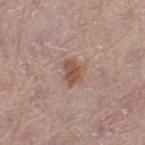Recorded during total-body skin imaging; not selected for excision or biopsy.
On the right thigh.
Imaged with white-light lighting.
Longest diameter approximately 3 mm.
A roughly 15 mm field-of-view crop from a total-body skin photograph.
A female subject, aged 63 to 67.
An algorithmic analysis of the crop reported border irregularity of about 3 on a 0–10 scale, internal color variation of about 2.5 on a 0–10 scale, and radial color variation of about 0.5. It also reported an automated nevus-likeness rating near 75 out of 100.